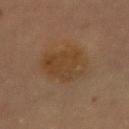Part of a total-body skin-imaging series; this lesion was reviewed on a skin check and was not flagged for biopsy.
Automated image analysis of the tile measured a detector confidence of about 100 out of 100 that the crop contains a lesion.
Measured at roughly 5.5 mm in maximum diameter.
The tile uses cross-polarized illumination.
The patient is a female aged approximately 60.
A region of skin cropped from a whole-body photographic capture, roughly 15 mm wide.
The lesion is located on the abdomen.